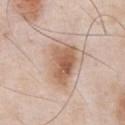A lesion tile, about 15 mm wide, cut from a 3D total-body photograph.
The patient is a male roughly 55 years of age.
This is a white-light tile.
The total-body-photography lesion software estimated a border-irregularity index near 3/10, a color-variation rating of about 7.5/10, and radial color variation of about 2.5. The analysis additionally found an automated nevus-likeness rating near 90 out of 100 and lesion-presence confidence of about 100/100.
The lesion is on the chest.
Approximately 6 mm at its widest.